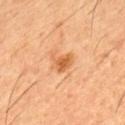Imaged during a routine full-body skin examination; the lesion was not biopsied and no histopathology is available.